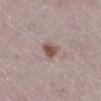| key | value |
|---|---|
| follow-up | imaged on a skin check; not biopsied |
| patient | female, about 50 years old |
| image source | ~15 mm crop, total-body skin-cancer survey |
| anatomic site | the right lower leg |
| image-analysis metrics | a border-irregularity rating of about 3/10 and internal color variation of about 3 on a 0–10 scale |
| illumination | white-light illumination |